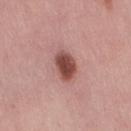Impression: Captured during whole-body skin photography for melanoma surveillance; the lesion was not biopsied. Image and clinical context: This image is a 15 mm lesion crop taken from a total-body photograph. On the right thigh. A female subject in their mid- to late 40s. About 3.5 mm across. Imaged with white-light lighting.